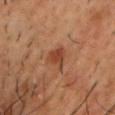Q: Was a biopsy performed?
A: no biopsy performed (imaged during a skin exam)
Q: Illumination type?
A: cross-polarized illumination
Q: Who is the patient?
A: male, about 50 years old
Q: What is the lesion's diameter?
A: ≈3 mm
Q: How was this image acquired?
A: ~15 mm crop, total-body skin-cancer survey
Q: Where on the body is the lesion?
A: the chest
Q: What did automated image analysis measure?
A: an area of roughly 5 mm², an outline eccentricity of about 0.7 (0 = round, 1 = elongated), and a shape-asymmetry score of about 0.35 (0 = symmetric); a lesion-to-skin contrast of about 7 (normalized; higher = more distinct); a border-irregularity rating of about 3/10, internal color variation of about 2.5 on a 0–10 scale, and peripheral color asymmetry of about 1; a classifier nevus-likeness of about 65/100 and a lesion-detection confidence of about 100/100